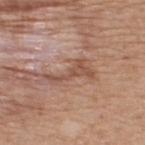Case summary:
- notes — catalogued during a skin exam; not biopsied